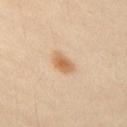Q: Was a biopsy performed?
A: imaged on a skin check; not biopsied
Q: What kind of image is this?
A: ~15 mm tile from a whole-body skin photo
Q: What did automated image analysis measure?
A: a lesion area of about 5 mm², an eccentricity of roughly 0.8, and two-axis asymmetry of about 0.25; a lesion color around L≈65 a*≈20 b*≈37 in CIELAB, about 11 CIELAB-L* units darker than the surrounding skin, and a lesion-to-skin contrast of about 7.5 (normalized; higher = more distinct); border irregularity of about 2.5 on a 0–10 scale, a color-variation rating of about 3/10, and a peripheral color-asymmetry measure near 1; an automated nevus-likeness rating near 95 out of 100 and a lesion-detection confidence of about 100/100
Q: Where on the body is the lesion?
A: the right upper arm
Q: Lesion size?
A: ~3 mm (longest diameter)
Q: What are the patient's age and sex?
A: male, in their 30s